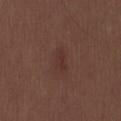workup: imaged on a skin check; not biopsied
lesion size: about 3 mm
anatomic site: the lower back
subject: male, approximately 30 years of age
tile lighting: white-light illumination
TBP lesion metrics: an average lesion color of about L≈32 a*≈20 b*≈21 (CIELAB), roughly 5 lightness units darker than nearby skin, and a normalized lesion–skin contrast near 5; a border-irregularity rating of about 3/10, a color-variation rating of about 1.5/10, and radial color variation of about 0.5
image source: 15 mm crop, total-body photography